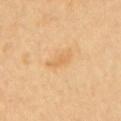No biopsy was performed on this lesion — it was imaged during a full skin examination and was not determined to be concerning. A region of skin cropped from a whole-body photographic capture, roughly 15 mm wide. Approximately 3.5 mm at its widest. This is a cross-polarized tile. On the arm. Automated image analysis of the tile measured a normalized lesion–skin contrast near 5. It also reported a nevus-likeness score of about 0/100 and lesion-presence confidence of about 100/100. The subject is a female in their 70s.